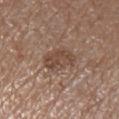Impression: This lesion was catalogued during total-body skin photography and was not selected for biopsy. Context: The tile uses white-light illumination. A 15 mm close-up extracted from a 3D total-body photography capture. A male patient, in their mid-50s. Measured at roughly 4.5 mm in maximum diameter. The lesion is on the right upper arm. The total-body-photography lesion software estimated an average lesion color of about L≈45 a*≈17 b*≈26 (CIELAB), about 9 CIELAB-L* units darker than the surrounding skin, and a lesion-to-skin contrast of about 7 (normalized; higher = more distinct). The software also gave a within-lesion color-variation index near 3/10 and peripheral color asymmetry of about 1.